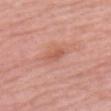biopsy status: total-body-photography surveillance lesion; no biopsy | patient: female, about 60 years old | size: ≈3 mm | imaging modality: ~15 mm tile from a whole-body skin photo | site: the head or neck.